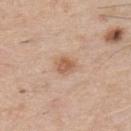Recorded during total-body skin imaging; not selected for excision or biopsy. A male patient, aged 58–62. From the chest. About 2.5 mm across. Cropped from a whole-body photographic skin survey; the tile spans about 15 mm.